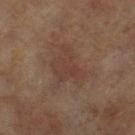biopsy status: imaged on a skin check; not biopsied
patient: female, about 60 years old
location: the left thigh
lesion diameter: ~4.5 mm (longest diameter)
image: ~15 mm tile from a whole-body skin photo